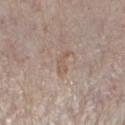workup: total-body-photography surveillance lesion; no biopsy
acquisition: ~15 mm tile from a whole-body skin photo
diameter: ~3 mm (longest diameter)
subject: female, roughly 55 years of age
body site: the left lower leg
illumination: white-light illumination